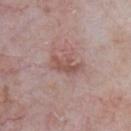Part of a total-body skin-imaging series; this lesion was reviewed on a skin check and was not flagged for biopsy. This is a white-light tile. A male subject, aged 63 to 67. The lesion is on the chest. About 3.5 mm across. A lesion tile, about 15 mm wide, cut from a 3D total-body photograph.